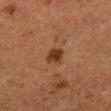workup — total-body-photography surveillance lesion; no biopsy | subject — female, aged 48 to 52 | site — the leg | image source — 15 mm crop, total-body photography | TBP lesion metrics — an area of roughly 4.5 mm², an outline eccentricity of about 0.7 (0 = round, 1 = elongated), and a shape-asymmetry score of about 0.2 (0 = symmetric); border irregularity of about 1.5 on a 0–10 scale, a within-lesion color-variation index near 2.5/10, and a peripheral color-asymmetry measure near 1 | diameter — about 2.5 mm | lighting — cross-polarized illumination.